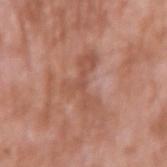<tbp_lesion>
<biopsy_status>not biopsied; imaged during a skin examination</biopsy_status>
<image>
  <source>total-body photography crop</source>
  <field_of_view_mm>15</field_of_view_mm>
</image>
<site>right upper arm</site>
<lighting>white-light</lighting>
<patient>
  <sex>male</sex>
  <age_approx>60</age_approx>
</patient>
<lesion_size>
  <long_diameter_mm_approx>6.0</long_diameter_mm_approx>
</lesion_size>
</tbp_lesion>